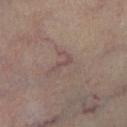<record>
  <biopsy_status>not biopsied; imaged during a skin examination</biopsy_status>
  <lesion_size>
    <long_diameter_mm_approx>2.5</long_diameter_mm_approx>
  </lesion_size>
  <image>
    <source>total-body photography crop</source>
    <field_of_view_mm>15</field_of_view_mm>
  </image>
  <site>right lower leg</site>
  <patient>
    <sex>male</sex>
    <age_approx>70</age_approx>
  </patient>
</record>